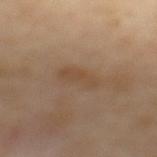image:
  source: total-body photography crop
  field_of_view_mm: 15
automated_metrics:
  vs_skin_darker_L: 6.0
  vs_skin_contrast_norm: 5.5
  color_variation_0_10: 2.0
  peripheral_color_asymmetry: 0.5
patient:
  sex: male
  age_approx: 65
lesion_size:
  long_diameter_mm_approx: 4.0
site: mid back
lighting: cross-polarized